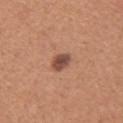Clinical impression: Captured during whole-body skin photography for melanoma surveillance; the lesion was not biopsied. Image and clinical context: A region of skin cropped from a whole-body photographic capture, roughly 15 mm wide. Located on the upper back. About 2.5 mm across. A female subject, aged 38–42.